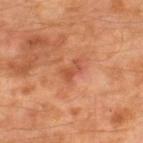Assessment: The lesion was photographed on a routine skin check and not biopsied; there is no pathology result. Acquisition and patient details: Captured under cross-polarized illumination. Automated tile analysis of the lesion measured a lesion color around L≈49 a*≈28 b*≈35 in CIELAB and about 8 CIELAB-L* units darker than the surrounding skin. The analysis additionally found a border-irregularity rating of about 5.5/10, internal color variation of about 0 on a 0–10 scale, and peripheral color asymmetry of about 0. A male subject aged 58 to 62. From the right lower leg. Cropped from a whole-body photographic skin survey; the tile spans about 15 mm. Longest diameter approximately 2.5 mm.